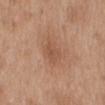Context:
An algorithmic analysis of the crop reported a lesion area of about 6.5 mm² and an eccentricity of roughly 0.6. The software also gave a lesion color around L≈53 a*≈21 b*≈31 in CIELAB. And it measured a nevus-likeness score of about 5/100 and a detector confidence of about 100 out of 100 that the crop contains a lesion. A female subject about 40 years old. A region of skin cropped from a whole-body photographic capture, roughly 15 mm wide. Approximately 3 mm at its widest. From the back.